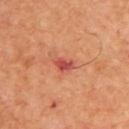<lesion>
<biopsy_status>not biopsied; imaged during a skin examination</biopsy_status>
<patient>
  <sex>male</sex>
  <age_approx>65</age_approx>
</patient>
<lighting>cross-polarized</lighting>
<image>
  <source>total-body photography crop</source>
  <field_of_view_mm>15</field_of_view_mm>
</image>
<site>upper back</site>
<lesion_size>
  <long_diameter_mm_approx>2.5</long_diameter_mm_approx>
</lesion_size>
</lesion>